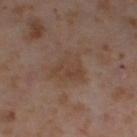Findings:
– follow-up — catalogued during a skin exam; not biopsied
– diameter — ≈4.5 mm
– TBP lesion metrics — a normalized lesion–skin contrast near 5.5; radial color variation of about 1; a classifier nevus-likeness of about 0/100 and a detector confidence of about 100 out of 100 that the crop contains a lesion
– location — the right thigh
– image — ~15 mm tile from a whole-body skin photo
– subject — female, aged 53 to 57
– lighting — cross-polarized